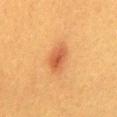Impression: The lesion was photographed on a routine skin check and not biopsied; there is no pathology result. Clinical summary: The lesion-visualizer software estimated a mean CIELAB color near L≈48 a*≈24 b*≈36 and a normalized border contrast of about 7.5. It also reported border irregularity of about 2 on a 0–10 scale, a within-lesion color-variation index near 3.5/10, and a peripheral color-asymmetry measure near 1. A female patient, approximately 40 years of age. A roughly 15 mm field-of-view crop from a total-body skin photograph. Located on the mid back. Captured under cross-polarized illumination. Longest diameter approximately 4 mm.